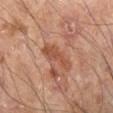Q: How was the tile lit?
A: cross-polarized
Q: Lesion size?
A: ~4.5 mm (longest diameter)
Q: What is the anatomic site?
A: the right lower leg
Q: What is the imaging modality?
A: 15 mm crop, total-body photography
Q: Who is the patient?
A: male, approximately 55 years of age